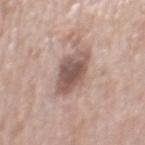{
  "biopsy_status": "not biopsied; imaged during a skin examination",
  "site": "mid back",
  "lesion_size": {
    "long_diameter_mm_approx": 5.0
  },
  "patient": {
    "sex": "male",
    "age_approx": 70
  },
  "image": {
    "source": "total-body photography crop",
    "field_of_view_mm": 15
  }
}